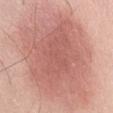Captured during whole-body skin photography for melanoma surveillance; the lesion was not biopsied. This image is a 15 mm lesion crop taken from a total-body photograph. Measured at roughly 10.5 mm in maximum diameter. A male patient about 70 years old. Located on the abdomen. Captured under white-light illumination.